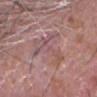Assessment: This lesion was catalogued during total-body skin photography and was not selected for biopsy. Background: The lesion-visualizer software estimated an area of roughly 14 mm², an eccentricity of roughly 0.6, and two-axis asymmetry of about 0.65. The analysis additionally found about 6 CIELAB-L* units darker than the surrounding skin and a lesion-to-skin contrast of about 4.5 (normalized; higher = more distinct). The software also gave radial color variation of about 1.5. The tile uses white-light illumination. Approximately 6 mm at its widest. The lesion is on the head or neck. Cropped from a total-body skin-imaging series; the visible field is about 15 mm. The subject is a male about 80 years old.